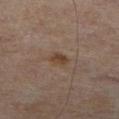biopsy status: total-body-photography surveillance lesion; no biopsy
imaging modality: total-body-photography crop, ~15 mm field of view
subject: male, in their mid- to late 60s
automated metrics: a nevus-likeness score of about 70/100 and a lesion-detection confidence of about 100/100
body site: the right lower leg